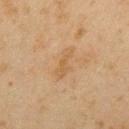biopsy_status: not biopsied; imaged during a skin examination
image:
  source: total-body photography crop
  field_of_view_mm: 15
lighting: cross-polarized
lesion_size:
  long_diameter_mm_approx: 4.0
site: arm
patient:
  sex: male
  age_approx: 45
automated_metrics:
  area_mm2_approx: 5.5
  eccentricity: 0.9
  cielab_L: 47
  cielab_a: 15
  cielab_b: 32
  vs_skin_darker_L: 5.0
  vs_skin_contrast_norm: 5.0
  border_irregularity_0_10: 4.0
  color_variation_0_10: 1.5
  nevus_likeness_0_100: 0
  lesion_detection_confidence_0_100: 100